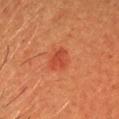follow-up=total-body-photography surveillance lesion; no biopsy | illumination=cross-polarized | subject=male, approximately 40 years of age | image source=total-body-photography crop, ~15 mm field of view | anatomic site=the head or neck | diameter=about 3.5 mm.